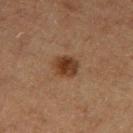Recorded during total-body skin imaging; not selected for excision or biopsy. The lesion's longest dimension is about 3 mm. A close-up tile cropped from a whole-body skin photograph, about 15 mm across. The tile uses cross-polarized illumination. A male patient, aged approximately 75. From the left thigh.